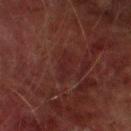follow-up: imaged on a skin check; not biopsied | lesion size: about 4 mm | location: the left thigh | image: ~15 mm tile from a whole-body skin photo | subject: male, approximately 75 years of age.